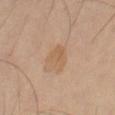{
  "site": "right thigh",
  "patient": {
    "sex": "female",
    "age_approx": 60
  },
  "lighting": "cross-polarized",
  "image": {
    "source": "total-body photography crop",
    "field_of_view_mm": 15
  },
  "automated_metrics": {
    "area_mm2_approx": 5.0,
    "eccentricity": 0.55,
    "cielab_L": 59,
    "cielab_a": 18,
    "cielab_b": 34,
    "vs_skin_darker_L": 6.0,
    "color_variation_0_10": 1.5,
    "peripheral_color_asymmetry": 0.5
  },
  "lesion_size": {
    "long_diameter_mm_approx": 3.0
  }
}